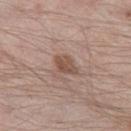Impression:
Imaged during a routine full-body skin examination; the lesion was not biopsied and no histopathology is available.